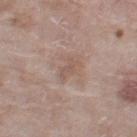Assessment: No biopsy was performed on this lesion — it was imaged during a full skin examination and was not determined to be concerning. Image and clinical context: Located on the left thigh. The tile uses white-light illumination. A 15 mm close-up tile from a total-body photography series done for melanoma screening. Approximately 2.5 mm at its widest. A female subject roughly 75 years of age.